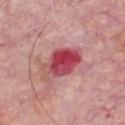Assessment:
The lesion was photographed on a routine skin check and not biopsied; there is no pathology result.
Background:
A 15 mm close-up extracted from a 3D total-body photography capture. The lesion is located on the chest. Measured at roughly 5.5 mm in maximum diameter. This is a white-light tile. A male subject approximately 65 years of age.